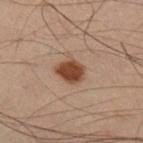Part of a total-body skin-imaging series; this lesion was reviewed on a skin check and was not flagged for biopsy.
A roughly 15 mm field-of-view crop from a total-body skin photograph.
The lesion is located on the right lower leg.
The patient is a male aged 53–57.